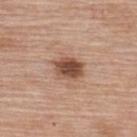Clinical impression: Recorded during total-body skin imaging; not selected for excision or biopsy. Acquisition and patient details: This image is a 15 mm lesion crop taken from a total-body photograph. The total-body-photography lesion software estimated an area of roughly 7.5 mm² and two-axis asymmetry of about 0.15. The analysis additionally found an average lesion color of about L≈49 a*≈21 b*≈29 (CIELAB) and a lesion–skin lightness drop of about 15. From the upper back. About 3.5 mm across. The patient is a female aged around 65.